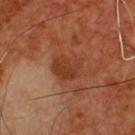Image and clinical context:
About 3.5 mm across. A male subject, about 55 years old. On the chest. A roughly 15 mm field-of-view crop from a total-body skin photograph. Imaged with cross-polarized lighting.